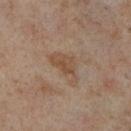No biopsy was performed on this lesion — it was imaged during a full skin examination and was not determined to be concerning.
Captured under cross-polarized illumination.
A female subject, approximately 50 years of age.
On the leg.
A 15 mm close-up tile from a total-body photography series done for melanoma screening.
Longest diameter approximately 4.5 mm.